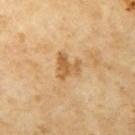Assessment: Captured during whole-body skin photography for melanoma surveillance; the lesion was not biopsied. Image and clinical context: Imaged with cross-polarized lighting. An algorithmic analysis of the crop reported an area of roughly 5.5 mm² and a symmetry-axis asymmetry near 0.55. It also reported an average lesion color of about L≈61 a*≈20 b*≈43 (CIELAB) and a lesion–skin lightness drop of about 11. The patient is a female aged 68–72. On the left upper arm. Longest diameter approximately 3 mm. A 15 mm crop from a total-body photograph taken for skin-cancer surveillance.